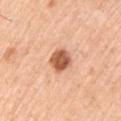| key | value |
|---|---|
| follow-up | no biopsy performed (imaged during a skin exam) |
| body site | the left upper arm |
| image source | 15 mm crop, total-body photography |
| subject | male, approximately 55 years of age |